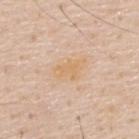biopsy_status: not biopsied; imaged during a skin examination
image:
  source: total-body photography crop
  field_of_view_mm: 15
lesion_size:
  long_diameter_mm_approx: 4.0
patient:
  sex: male
  age_approx: 60
site: back
lighting: white-light